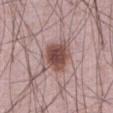Q: Is there a histopathology result?
A: total-body-photography surveillance lesion; no biopsy
Q: What lighting was used for the tile?
A: white-light
Q: What are the patient's age and sex?
A: male, in their mid- to late 60s
Q: What is the anatomic site?
A: the abdomen
Q: How was this image acquired?
A: 15 mm crop, total-body photography
Q: How large is the lesion?
A: ~5 mm (longest diameter)